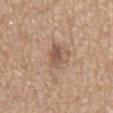Recorded during total-body skin imaging; not selected for excision or biopsy.
A 15 mm close-up extracted from a 3D total-body photography capture.
The patient is a male approximately 70 years of age.
Automated tile analysis of the lesion measured a lesion area of about 6 mm², a shape eccentricity near 0.85, and two-axis asymmetry of about 0.25. The software also gave an average lesion color of about L≈54 a*≈18 b*≈28 (CIELAB), a lesion–skin lightness drop of about 10, and a lesion-to-skin contrast of about 7 (normalized; higher = more distinct). The software also gave border irregularity of about 3 on a 0–10 scale and a peripheral color-asymmetry measure near 1.5. The analysis additionally found a nevus-likeness score of about 0/100 and a detector confidence of about 100 out of 100 that the crop contains a lesion.
From the mid back.